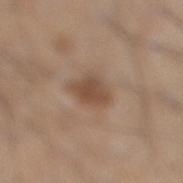{
  "biopsy_status": "not biopsied; imaged during a skin examination",
  "image": {
    "source": "total-body photography crop",
    "field_of_view_mm": 15
  },
  "site": "right lower leg",
  "lighting": "white-light",
  "patient": {
    "sex": "male",
    "age_approx": 45
  }
}